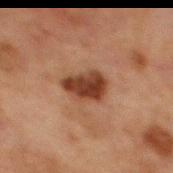biopsy_status: not biopsied; imaged during a skin examination
automated_metrics:
  area_mm2_approx: 10.0
  eccentricity: 0.75
  shape_asymmetry: 0.2
  lesion_detection_confidence_0_100: 100
patient:
  sex: male
  age_approx: 65
lesion_size:
  long_diameter_mm_approx: 4.5
site: chest
image:
  source: total-body photography crop
  field_of_view_mm: 15
lighting: cross-polarized